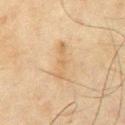Clinical impression: Captured during whole-body skin photography for melanoma surveillance; the lesion was not biopsied. Image and clinical context: A close-up tile cropped from a whole-body skin photograph, about 15 mm across. The lesion is on the front of the torso. Imaged with cross-polarized lighting. The lesion's longest dimension is about 4.5 mm. A male patient roughly 45 years of age.